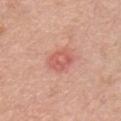Clinical impression: Part of a total-body skin-imaging series; this lesion was reviewed on a skin check and was not flagged for biopsy. Clinical summary: A lesion tile, about 15 mm wide, cut from a 3D total-body photograph. This is a white-light tile. The lesion-visualizer software estimated a lesion area of about 6.5 mm² and a shape eccentricity near 0.65. And it measured an average lesion color of about L≈59 a*≈29 b*≈28 (CIELAB) and a lesion–skin lightness drop of about 9. The analysis additionally found a classifier nevus-likeness of about 0/100 and a lesion-detection confidence of about 100/100. Approximately 3 mm at its widest. Located on the front of the torso. The subject is a male aged around 50.